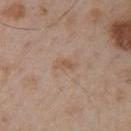Imaged during a routine full-body skin examination; the lesion was not biopsied and no histopathology is available. A male patient, aged approximately 55. A 15 mm close-up extracted from a 3D total-body photography capture. Automated image analysis of the tile measured a classifier nevus-likeness of about 0/100 and lesion-presence confidence of about 100/100. Located on the right upper arm. The tile uses white-light illumination. The recorded lesion diameter is about 2.5 mm.